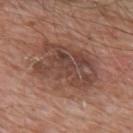biopsy_status: not biopsied; imaged during a skin examination
site: back
lighting: white-light
patient:
  sex: male
  age_approx: 80
image:
  source: total-body photography crop
  field_of_view_mm: 15
lesion_size:
  long_diameter_mm_approx: 7.5